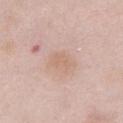{
  "image": {
    "source": "total-body photography crop",
    "field_of_view_mm": 15
  },
  "automated_metrics": {
    "area_mm2_approx": 5.5,
    "eccentricity": 0.7,
    "shape_asymmetry": 0.3,
    "border_irregularity_0_10": 3.0,
    "peripheral_color_asymmetry": 0.5
  },
  "patient": {
    "sex": "female",
    "age_approx": 65
  },
  "site": "chest"
}